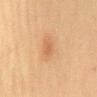Part of a total-body skin-imaging series; this lesion was reviewed on a skin check and was not flagged for biopsy.
Measured at roughly 2.5 mm in maximum diameter.
The patient is a female aged around 55.
From the mid back.
A close-up tile cropped from a whole-body skin photograph, about 15 mm across.
An algorithmic analysis of the crop reported a lesion area of about 3 mm², a shape eccentricity near 0.85, and two-axis asymmetry of about 0.35. And it measured border irregularity of about 3 on a 0–10 scale and peripheral color asymmetry of about 0.5. It also reported a nevus-likeness score of about 10/100.